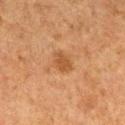<lesion>
  <biopsy_status>not biopsied; imaged during a skin examination</biopsy_status>
  <lesion_size>
    <long_diameter_mm_approx>3.0</long_diameter_mm_approx>
  </lesion_size>
  <patient>
    <sex>male</sex>
    <age_approx>75</age_approx>
  </patient>
  <image>
    <source>total-body photography crop</source>
    <field_of_view_mm>15</field_of_view_mm>
  </image>
  <lighting>cross-polarized</lighting>
  <site>leg</site>
</lesion>